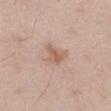Assessment:
Captured during whole-body skin photography for melanoma surveillance; the lesion was not biopsied.
Acquisition and patient details:
Cropped from a whole-body photographic skin survey; the tile spans about 15 mm. A male patient, aged 58 to 62. The total-body-photography lesion software estimated a lesion area of about 4.5 mm², an outline eccentricity of about 0.35 (0 = round, 1 = elongated), and two-axis asymmetry of about 0.4. It also reported a border-irregularity rating of about 4/10. The analysis additionally found an automated nevus-likeness rating near 10 out of 100 and lesion-presence confidence of about 100/100. This is a white-light tile. The lesion's longest dimension is about 2.5 mm. From the left thigh.